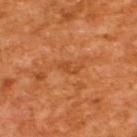The lesion was photographed on a routine skin check and not biopsied; there is no pathology result.
A male patient, aged 63 to 67.
The lesion is located on the upper back.
The lesion-visualizer software estimated a lesion area of about 2.5 mm², an outline eccentricity of about 0.9 (0 = round, 1 = elongated), and a symmetry-axis asymmetry near 0.45. The software also gave a lesion–skin lightness drop of about 7 and a normalized border contrast of about 5. The analysis additionally found border irregularity of about 5.5 on a 0–10 scale and a peripheral color-asymmetry measure near 0.
A 15 mm close-up extracted from a 3D total-body photography capture.
Longest diameter approximately 2.5 mm.
Captured under cross-polarized illumination.